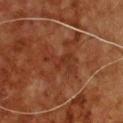No biopsy was performed on this lesion — it was imaged during a full skin examination and was not determined to be concerning.
A male patient about 60 years old.
The lesion is on the chest.
Measured at roughly 5 mm in maximum diameter.
A lesion tile, about 15 mm wide, cut from a 3D total-body photograph.
Automated image analysis of the tile measured a lesion area of about 8.5 mm² and a shape eccentricity near 0.9. It also reported an automated nevus-likeness rating near 0 out of 100 and a detector confidence of about 95 out of 100 that the crop contains a lesion.